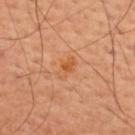– tile lighting · cross-polarized illumination
– image source · 15 mm crop, total-body photography
– subject · male, approximately 60 years of age
– anatomic site · the upper back
– lesion diameter · ≈3 mm